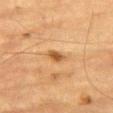A male subject, aged around 85 or older. The total-body-photography lesion software estimated an average lesion color of about L≈48 a*≈21 b*≈37 (CIELAB), about 12 CIELAB-L* units darker than the surrounding skin, and a normalized lesion–skin contrast near 8.5. It also reported border irregularity of about 3 on a 0–10 scale, internal color variation of about 2 on a 0–10 scale, and peripheral color asymmetry of about 0.5. Approximately 2.5 mm at its widest. Imaged with cross-polarized lighting. The lesion is on the leg. A 15 mm crop from a total-body photograph taken for skin-cancer surveillance.